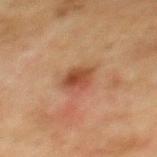Image and clinical context: A male patient, aged approximately 75. The recorded lesion diameter is about 2.5 mm. Imaged with cross-polarized lighting. Cropped from a total-body skin-imaging series; the visible field is about 15 mm. The lesion-visualizer software estimated a border-irregularity index near 2/10 and a color-variation rating of about 4/10. From the mid back.